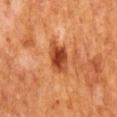A close-up tile cropped from a whole-body skin photograph, about 15 mm across. A male subject, approximately 65 years of age. Located on the mid back.